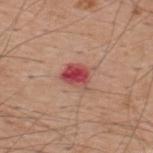notes: catalogued during a skin exam; not biopsied | anatomic site: the upper back | tile lighting: white-light | lesion size: ≈3.5 mm | acquisition: 15 mm crop, total-body photography | patient: male, roughly 60 years of age.